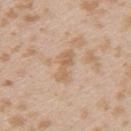workup: no biopsy performed (imaged during a skin exam) | image source: total-body-photography crop, ~15 mm field of view | diameter: about 4 mm | body site: the left upper arm | patient: female, about 25 years old | lighting: white-light.